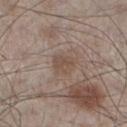Q: Was a biopsy performed?
A: catalogued during a skin exam; not biopsied
Q: What is the anatomic site?
A: the left lower leg
Q: What are the patient's age and sex?
A: male, about 60 years old
Q: What is the imaging modality?
A: total-body-photography crop, ~15 mm field of view
Q: Illumination type?
A: white-light illumination
Q: What did automated image analysis measure?
A: a lesion area of about 5.5 mm², a shape eccentricity near 0.65, and a shape-asymmetry score of about 0.25 (0 = symmetric); about 7 CIELAB-L* units darker than the surrounding skin and a lesion-to-skin contrast of about 5.5 (normalized; higher = more distinct); a border-irregularity index near 2.5/10, a within-lesion color-variation index near 2/10, and radial color variation of about 0.5; a nevus-likeness score of about 0/100 and a detector confidence of about 100 out of 100 that the crop contains a lesion
Q: What is the lesion's diameter?
A: ~3 mm (longest diameter)